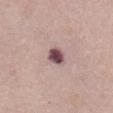* notes · no biopsy performed (imaged during a skin exam)
* subject · female, in their mid-60s
* imaging modality · ~15 mm tile from a whole-body skin photo
* diameter · ≈3 mm
* automated lesion analysis · a footprint of about 5 mm², an eccentricity of roughly 0.6, and a symmetry-axis asymmetry near 0.2
* lighting · white-light illumination
* site · the front of the torso